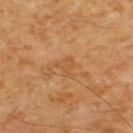Assessment:
Recorded during total-body skin imaging; not selected for excision or biopsy.
Clinical summary:
Imaged with cross-polarized lighting. A lesion tile, about 15 mm wide, cut from a 3D total-body photograph. About 2.5 mm across. The subject is a male aged 58 to 62. An algorithmic analysis of the crop reported an automated nevus-likeness rating near 0 out of 100 and a lesion-detection confidence of about 100/100. From the upper back.